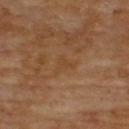{
  "biopsy_status": "not biopsied; imaged during a skin examination",
  "lesion_size": {
    "long_diameter_mm_approx": 2.5
  },
  "site": "upper back",
  "patient": {
    "sex": "male",
    "age_approx": 70
  },
  "automated_metrics": {
    "area_mm2_approx": 3.0,
    "eccentricity": 0.65,
    "shape_asymmetry": 0.6,
    "border_irregularity_0_10": 6.0,
    "color_variation_0_10": 0.5,
    "peripheral_color_asymmetry": 0.0
  },
  "image": {
    "source": "total-body photography crop",
    "field_of_view_mm": 15
  },
  "lighting": "cross-polarized"
}